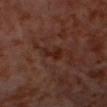notes=imaged on a skin check; not biopsied.